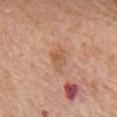Imaged during a routine full-body skin examination; the lesion was not biopsied and no histopathology is available. From the upper back. The subject is a female approximately 65 years of age. Cropped from a whole-body photographic skin survey; the tile spans about 15 mm. Measured at roughly 2.5 mm in maximum diameter.